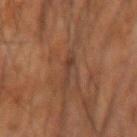Captured during whole-body skin photography for melanoma surveillance; the lesion was not biopsied. A 15 mm close-up extracted from a 3D total-body photography capture. Imaged with cross-polarized lighting. The patient is a male about 60 years old. The lesion is on the right forearm. About 3.5 mm across.